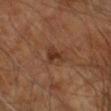Assessment: The lesion was tiled from a total-body skin photograph and was not biopsied.